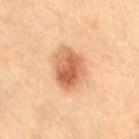{"biopsy_status": "not biopsied; imaged during a skin examination", "patient": {"sex": "female", "age_approx": 55}, "site": "right thigh", "lighting": "cross-polarized", "image": {"source": "total-body photography crop", "field_of_view_mm": 15}}